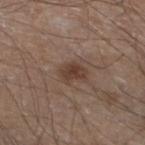A male subject in their mid- to late 40s. A 15 mm crop from a total-body photograph taken for skin-cancer surveillance. The lesion is located on the left lower leg. An algorithmic analysis of the crop reported a footprint of about 5.5 mm², a shape eccentricity near 0.65, and two-axis asymmetry of about 0.3. And it measured a lesion color around L≈39 a*≈17 b*≈24 in CIELAB and roughly 9 lightness units darker than nearby skin. And it measured internal color variation of about 2.5 on a 0–10 scale and radial color variation of about 0.5. The software also gave a nevus-likeness score of about 90/100 and a lesion-detection confidence of about 100/100.